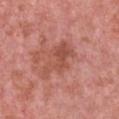Recorded during total-body skin imaging; not selected for excision or biopsy.
Automated image analysis of the tile measured a mean CIELAB color near L≈51 a*≈27 b*≈29, roughly 8 lightness units darker than nearby skin, and a normalized lesion–skin contrast near 6.
Approximately 5.5 mm at its widest.
Captured under white-light illumination.
The patient is a female in their 40s.
The lesion is located on the front of the torso.
A roughly 15 mm field-of-view crop from a total-body skin photograph.